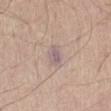Q: Is there a histopathology result?
A: catalogued during a skin exam; not biopsied
Q: What is the lesion's diameter?
A: about 3 mm
Q: What is the anatomic site?
A: the front of the torso
Q: Patient demographics?
A: male, approximately 65 years of age
Q: What kind of image is this?
A: total-body-photography crop, ~15 mm field of view
Q: Illumination type?
A: white-light illumination